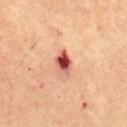<tbp_lesion>
<biopsy_status>not biopsied; imaged during a skin examination</biopsy_status>
<site>mid back</site>
<patient>
  <sex>male</sex>
  <age_approx>65</age_approx>
</patient>
<image>
  <source>total-body photography crop</source>
  <field_of_view_mm>15</field_of_view_mm>
</image>
<lighting>cross-polarized</lighting>
<lesion_size>
  <long_diameter_mm_approx>3.0</long_diameter_mm_approx>
</lesion_size>
</tbp_lesion>